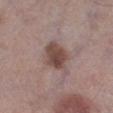Imaged during a routine full-body skin examination; the lesion was not biopsied and no histopathology is available.
From the left lower leg.
A male subject aged 53–57.
This image is a 15 mm lesion crop taken from a total-body photograph.